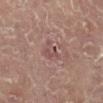Impression: The lesion was photographed on a routine skin check and not biopsied; there is no pathology result. Background: Approximately 3 mm at its widest. A 15 mm close-up tile from a total-body photography series done for melanoma screening. The lesion is located on the right leg. A female patient, aged approximately 80. Imaged with cross-polarized lighting.